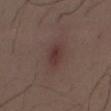follow-up: imaged on a skin check; not biopsied
image-analysis metrics: a shape eccentricity near 0.75 and a symmetry-axis asymmetry near 0.15; a border-irregularity index near 1.5/10, a within-lesion color-variation index near 3/10, and a peripheral color-asymmetry measure near 1; lesion-presence confidence of about 100/100
lighting: white-light
image source: ~15 mm tile from a whole-body skin photo
lesion size: ~3 mm (longest diameter)
body site: the mid back
subject: male, aged approximately 30